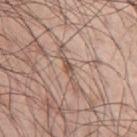Captured during whole-body skin photography for melanoma surveillance; the lesion was not biopsied. On the left upper arm. A male patient, in their mid- to late 40s. A close-up tile cropped from a whole-body skin photograph, about 15 mm across. Imaged with white-light lighting.